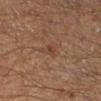Findings:
* workup — catalogued during a skin exam; not biopsied
* patient — male, about 45 years old
* image-analysis metrics — a lesion color around L≈34 a*≈15 b*≈24 in CIELAB and a normalized border contrast of about 4.5; internal color variation of about 2 on a 0–10 scale and radial color variation of about 0.5; a classifier nevus-likeness of about 0/100 and lesion-presence confidence of about 100/100
* image source — ~15 mm tile from a whole-body skin photo
* location — the leg